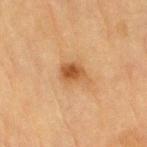The lesion was tiled from a total-body skin photograph and was not biopsied. A male patient about 85 years old or older. The lesion is located on the right upper arm. A roughly 15 mm field-of-view crop from a total-body skin photograph. An algorithmic analysis of the crop reported a footprint of about 6.5 mm². The software also gave a mean CIELAB color near L≈48 a*≈20 b*≈37 and a lesion-to-skin contrast of about 8 (normalized; higher = more distinct). The analysis additionally found border irregularity of about 3 on a 0–10 scale, a color-variation rating of about 4/10, and peripheral color asymmetry of about 1.5. The software also gave a classifier nevus-likeness of about 90/100 and lesion-presence confidence of about 100/100. The tile uses cross-polarized illumination. The recorded lesion diameter is about 3.5 mm.